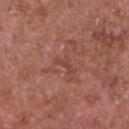Impression: Captured during whole-body skin photography for melanoma surveillance; the lesion was not biopsied. Background: The lesion is on the chest. Cropped from a total-body skin-imaging series; the visible field is about 15 mm. A male patient, about 65 years old. The recorded lesion diameter is about 2.5 mm.